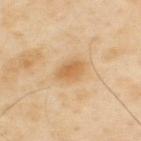notes: no biopsy performed (imaged during a skin exam); subject: male, aged around 55; site: the upper back; imaging modality: ~15 mm tile from a whole-body skin photo.